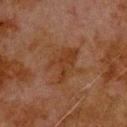workup: no biopsy performed (imaged during a skin exam)
image-analysis metrics: a shape eccentricity near 0.7; lesion-presence confidence of about 100/100
patient: male, aged around 80
image: 15 mm crop, total-body photography
anatomic site: the upper back
diameter: ≈5 mm